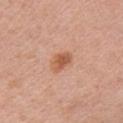biopsy status = catalogued during a skin exam; not biopsied | lesion size = ~3 mm (longest diameter) | acquisition = ~15 mm tile from a whole-body skin photo | TBP lesion metrics = a footprint of about 5 mm², an eccentricity of roughly 0.7, and two-axis asymmetry of about 0.3; a classifier nevus-likeness of about 75/100 | lighting = white-light | anatomic site = the chest | patient = female, aged 58–62.